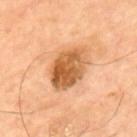Q: Was this lesion biopsied?
A: no biopsy performed (imaged during a skin exam)
Q: Who is the patient?
A: male, aged 63–67
Q: Lesion location?
A: the upper back
Q: Illumination type?
A: cross-polarized illumination
Q: What kind of image is this?
A: total-body-photography crop, ~15 mm field of view
Q: Lesion size?
A: about 5.5 mm
Q: What did automated image analysis measure?
A: a footprint of about 16 mm² and an eccentricity of roughly 0.75; a lesion color around L≈57 a*≈23 b*≈41 in CIELAB, about 15 CIELAB-L* units darker than the surrounding skin, and a normalized lesion–skin contrast near 9.5; border irregularity of about 2 on a 0–10 scale and a color-variation rating of about 7.5/10; a classifier nevus-likeness of about 5/100